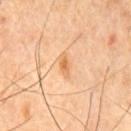Case summary:
• workup · imaged on a skin check; not biopsied
• lighting · cross-polarized illumination
• body site · the chest
• patient · male, aged approximately 65
• imaging modality · 15 mm crop, total-body photography